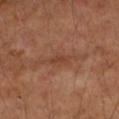No biopsy was performed on this lesion — it was imaged during a full skin examination and was not determined to be concerning. Longest diameter approximately 3 mm. Imaged with cross-polarized lighting. A close-up tile cropped from a whole-body skin photograph, about 15 mm across. The lesion is on the left forearm. The patient is a female in their 60s.